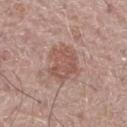Case summary:
– TBP lesion metrics — an eccentricity of roughly 0.5 and two-axis asymmetry of about 0.2; lesion-presence confidence of about 100/100
– location — the right thigh
– size — ≈4.5 mm
– image source — 15 mm crop, total-body photography
– subject — male, aged around 70
– lighting — white-light illumination